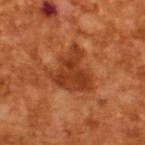{"biopsy_status": "not biopsied; imaged during a skin examination", "patient": {"sex": "male", "age_approx": 65}, "image": {"source": "total-body photography crop", "field_of_view_mm": 15}, "lighting": "cross-polarized", "lesion_size": {"long_diameter_mm_approx": 5.0}}